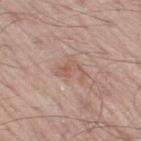Captured during whole-body skin photography for melanoma surveillance; the lesion was not biopsied.